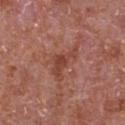No biopsy was performed on this lesion — it was imaged during a full skin examination and was not determined to be concerning.
Cropped from a whole-body photographic skin survey; the tile spans about 15 mm.
On the chest.
This is a white-light tile.
The total-body-photography lesion software estimated a footprint of about 7 mm² and a shape-asymmetry score of about 0.6 (0 = symmetric). The analysis additionally found a mean CIELAB color near L≈44 a*≈27 b*≈28 and a normalized lesion–skin contrast near 6.5. The software also gave border irregularity of about 7 on a 0–10 scale, a color-variation rating of about 2/10, and radial color variation of about 0.5. It also reported a nevus-likeness score of about 0/100 and lesion-presence confidence of about 100/100.
A male subject, about 65 years old.
Approximately 4.5 mm at its widest.